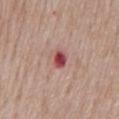{"biopsy_status": "not biopsied; imaged during a skin examination", "lighting": "white-light", "site": "mid back", "image": {"source": "total-body photography crop", "field_of_view_mm": 15}, "patient": {"sex": "male", "age_approx": 75}}